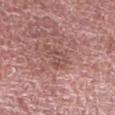biopsy status: catalogued during a skin exam; not biopsied
lesion size: ≈2.5 mm
automated metrics: a lesion color around L≈50 a*≈23 b*≈23 in CIELAB, roughly 6 lightness units darker than nearby skin, and a normalized lesion–skin contrast near 4.5; an automated nevus-likeness rating near 0 out of 100 and lesion-presence confidence of about 100/100
body site: the right forearm
tile lighting: white-light
image: ~15 mm tile from a whole-body skin photo
subject: female, aged 58–62